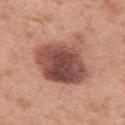Q: Is there a histopathology result?
A: total-body-photography surveillance lesion; no biopsy
Q: Automated lesion metrics?
A: an area of roughly 29 mm² and a shape eccentricity near 0.6; a classifier nevus-likeness of about 15/100
Q: What is the anatomic site?
A: the arm
Q: How large is the lesion?
A: about 7 mm
Q: Patient demographics?
A: female, in their 40s
Q: What kind of image is this?
A: ~15 mm crop, total-body skin-cancer survey
Q: How was the tile lit?
A: white-light